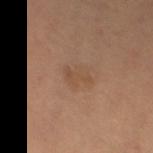No biopsy was performed on this lesion — it was imaged during a full skin examination and was not determined to be concerning. The recorded lesion diameter is about 3 mm. A female subject, approximately 50 years of age. Imaged with cross-polarized lighting. This image is a 15 mm lesion crop taken from a total-body photograph. The lesion is located on the left thigh.